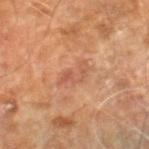Q: Lesion size?
A: about 4 mm
Q: What did automated image analysis measure?
A: a lesion color around L≈54 a*≈25 b*≈32 in CIELAB, roughly 7 lightness units darker than nearby skin, and a normalized lesion–skin contrast near 5; an automated nevus-likeness rating near 0 out of 100 and lesion-presence confidence of about 100/100
Q: What is the anatomic site?
A: the leg
Q: What are the patient's age and sex?
A: male, approximately 60 years of age
Q: What kind of image is this?
A: ~15 mm crop, total-body skin-cancer survey